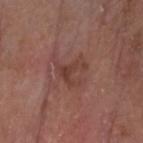Acquisition and patient details: The lesion is located on the head or neck. A 15 mm close-up extracted from a 3D total-body photography capture. A male subject roughly 80 years of age.